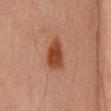follow-up: total-body-photography surveillance lesion; no biopsy
lighting: cross-polarized illumination
subject: male, aged around 70
diameter: ~4 mm (longest diameter)
imaging modality: total-body-photography crop, ~15 mm field of view
body site: the back